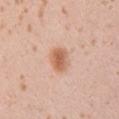{"biopsy_status": "not biopsied; imaged during a skin examination", "patient": {"sex": "female", "age_approx": 20}, "site": "arm", "automated_metrics": {"area_mm2_approx": 6.5, "eccentricity": 0.6, "nevus_likeness_0_100": 100, "lesion_detection_confidence_0_100": 100}, "image": {"source": "total-body photography crop", "field_of_view_mm": 15}}